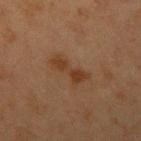workup: imaged on a skin check; not biopsied | subject: female, in their 40s | illumination: cross-polarized illumination | location: the left upper arm | lesion size: ≈5 mm | acquisition: total-body-photography crop, ~15 mm field of view.